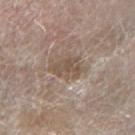Notes:
– notes: total-body-photography surveillance lesion; no biopsy
– image-analysis metrics: an outline eccentricity of about 0.75 (0 = round, 1 = elongated) and two-axis asymmetry of about 0.3; about 8 CIELAB-L* units darker than the surrounding skin and a normalized border contrast of about 6.5
– tile lighting: white-light illumination
– imaging modality: ~15 mm crop, total-body skin-cancer survey
– lesion diameter: ~4 mm (longest diameter)
– patient: male, roughly 80 years of age
– location: the right forearm